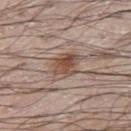No biopsy was performed on this lesion — it was imaged during a full skin examination and was not determined to be concerning. Longest diameter approximately 4.5 mm. On the leg. The patient is a male roughly 70 years of age. An algorithmic analysis of the crop reported a footprint of about 9 mm² and a shape-asymmetry score of about 0.25 (0 = symmetric). The analysis additionally found a mean CIELAB color near L≈50 a*≈16 b*≈26, about 11 CIELAB-L* units darker than the surrounding skin, and a normalized lesion–skin contrast near 8. The software also gave a nevus-likeness score of about 90/100 and a lesion-detection confidence of about 100/100. Cropped from a whole-body photographic skin survey; the tile spans about 15 mm.